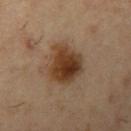The lesion was tiled from a total-body skin photograph and was not biopsied.
Captured under cross-polarized illumination.
The subject is a male aged 53–57.
A 15 mm crop from a total-body photograph taken for skin-cancer surveillance.
Longest diameter approximately 5 mm.
On the left upper arm.